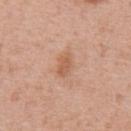Approximately 3 mm at its widest. Automated tile analysis of the lesion measured a lesion color around L≈59 a*≈22 b*≈33 in CIELAB. And it measured border irregularity of about 2 on a 0–10 scale and radial color variation of about 0.5. The patient is a male aged 53–57. A region of skin cropped from a whole-body photographic capture, roughly 15 mm wide. Located on the chest. Imaged with white-light lighting.